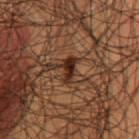notes: imaged on a skin check; not biopsied | patient: male, aged around 50 | image source: ~15 mm crop, total-body skin-cancer survey | image-analysis metrics: an automated nevus-likeness rating near 95 out of 100 and a lesion-detection confidence of about 85/100 | anatomic site: the mid back.